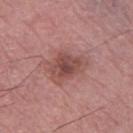The lesion was photographed on a routine skin check and not biopsied; there is no pathology result. The patient is a male aged 73–77. Cropped from a whole-body photographic skin survey; the tile spans about 15 mm. On the left thigh.